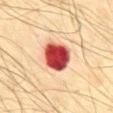Recorded during total-body skin imaging; not selected for excision or biopsy. The patient is a male in their mid- to late 70s. A close-up tile cropped from a whole-body skin photograph, about 15 mm across. An algorithmic analysis of the crop reported an area of roughly 12 mm², an eccentricity of roughly 0.65, and a shape-asymmetry score of about 0.15 (0 = symmetric). The software also gave a lesion color around L≈44 a*≈35 b*≈28 in CIELAB and about 24 CIELAB-L* units darker than the surrounding skin. The lesion is on the mid back. This is a cross-polarized tile. Approximately 4.5 mm at its widest.